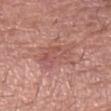follow-up: catalogued during a skin exam; not biopsied
body site: the right forearm
image source: total-body-photography crop, ~15 mm field of view
automated metrics: a mean CIELAB color near L≈54 a*≈25 b*≈25, roughly 7 lightness units darker than nearby skin, and a normalized lesion–skin contrast near 5
tile lighting: white-light illumination
patient: male, aged approximately 75